A male subject in their mid-50s, this image is a 15 mm lesion crop taken from a total-body photograph, from the head or neck — 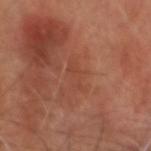Conclusion: Histopathology of the biopsied lesion showed a squamous cell carcinoma in situ (malignant).